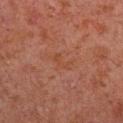{
  "image": {
    "source": "total-body photography crop",
    "field_of_view_mm": 15
  },
  "automated_metrics": {
    "eccentricity": 0.85,
    "shape_asymmetry": 0.5,
    "border_irregularity_0_10": 6.5,
    "peripheral_color_asymmetry": 0.0,
    "nevus_likeness_0_100": 0,
    "lesion_detection_confidence_0_100": 100
  },
  "site": "right upper arm",
  "patient": {
    "sex": "male",
    "age_approx": 30
  }
}